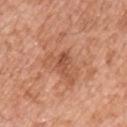• notes · imaged on a skin check; not biopsied